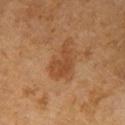Captured during whole-body skin photography for melanoma surveillance; the lesion was not biopsied. A 15 mm crop from a total-body photograph taken for skin-cancer surveillance. From the right upper arm. The total-body-photography lesion software estimated border irregularity of about 5.5 on a 0–10 scale and radial color variation of about 0.5. The software also gave a classifier nevus-likeness of about 5/100. The patient is a female in their 60s. Longest diameter approximately 4.5 mm.